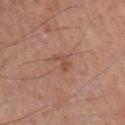Recorded during total-body skin imaging; not selected for excision or biopsy.